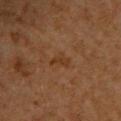  biopsy_status: not biopsied; imaged during a skin examination
  patient:
    sex: male
    age_approx: 60
  site: chest
  lesion_size:
    long_diameter_mm_approx: 3.0
  image:
    source: total-body photography crop
    field_of_view_mm: 15